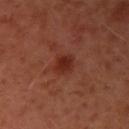notes=total-body-photography surveillance lesion; no biopsy | TBP lesion metrics=border irregularity of about 1.5 on a 0–10 scale, a within-lesion color-variation index near 2.5/10, and a peripheral color-asymmetry measure near 1; a classifier nevus-likeness of about 90/100 and a lesion-detection confidence of about 100/100 | acquisition=~15 mm tile from a whole-body skin photo | patient=female, aged 38 to 42 | tile lighting=cross-polarized | lesion size=~3 mm (longest diameter) | site=the right upper arm.